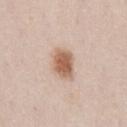Findings:
– body site — the chest
– imaging modality — 15 mm crop, total-body photography
– patient — male, aged 48 to 52
– automated lesion analysis — a mean CIELAB color near L≈60 a*≈20 b*≈30, about 14 CIELAB-L* units darker than the surrounding skin, and a normalized border contrast of about 9.5; a within-lesion color-variation index near 3/10 and a peripheral color-asymmetry measure near 1
– lesion size — about 3.5 mm